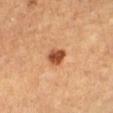- follow-up: total-body-photography surveillance lesion; no biopsy
- image source: ~15 mm crop, total-body skin-cancer survey
- image-analysis metrics: an outline eccentricity of about 0.5 (0 = round, 1 = elongated); a lesion color around L≈47 a*≈26 b*≈35 in CIELAB, roughly 15 lightness units darker than nearby skin, and a lesion-to-skin contrast of about 10.5 (normalized; higher = more distinct); a border-irregularity index near 2/10, a color-variation rating of about 3/10, and radial color variation of about 1
- patient: female, in their 60s
- lighting: cross-polarized illumination
- location: the left lower leg